A close-up tile cropped from a whole-body skin photograph, about 15 mm across; about 7.5 mm across; a female subject, aged approximately 40; this is a white-light tile; located on the left lower leg; An algorithmic analysis of the crop reported a lesion area of about 29 mm², an eccentricity of roughly 0.7, and a shape-asymmetry score of about 0.3 (0 = symmetric). It also reported an average lesion color of about L≈51 a*≈26 b*≈20 (CIELAB), a lesion–skin lightness drop of about 13, and a lesion-to-skin contrast of about 9 (normalized; higher = more distinct). And it measured a border-irregularity index near 3/10, internal color variation of about 8.5 on a 0–10 scale, and peripheral color asymmetry of about 2.5.
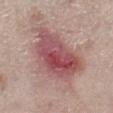<record>
  <diagnosis>
    <histopathology>superficial basal cell carcinoma</histopathology>
    <malignancy>malignant</malignancy>
    <taxonomic_path>Malignant; Malignant adnexal epithelial proliferations - Follicular; Basal cell carcinoma; Basal cell carcinoma, Superficial</taxonomic_path>
  </diagnosis>
</record>